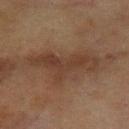Clinical impression: The lesion was tiled from a total-body skin photograph and was not biopsied. Context: On the arm. Cropped from a whole-body photographic skin survey; the tile spans about 15 mm. Approximately 8 mm at its widest. The tile uses cross-polarized illumination. Automated tile analysis of the lesion measured an area of roughly 18 mm², an outline eccentricity of about 0.9 (0 = round, 1 = elongated), and a shape-asymmetry score of about 0.55 (0 = symmetric). It also reported a lesion color around L≈35 a*≈16 b*≈25 in CIELAB and roughly 7 lightness units darker than nearby skin. A female patient, in their 60s.